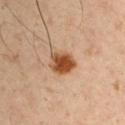Impression:
Captured during whole-body skin photography for melanoma surveillance; the lesion was not biopsied.
Background:
A lesion tile, about 15 mm wide, cut from a 3D total-body photograph. The lesion is located on the left upper arm. A male subject in their 50s.